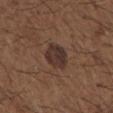Assessment: No biopsy was performed on this lesion — it was imaged during a full skin examination and was not determined to be concerning. Clinical summary: This is a white-light tile. About 3.5 mm across. On the right upper arm. A male patient roughly 50 years of age. A close-up tile cropped from a whole-body skin photograph, about 15 mm across.